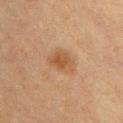workup: imaged on a skin check; not biopsied
patient: female, in their mid-50s
size: ≈4 mm
image-analysis metrics: a footprint of about 8.5 mm², a shape eccentricity near 0.7, and a symmetry-axis asymmetry near 0.25; an average lesion color of about L≈43 a*≈17 b*≈30 (CIELAB), a lesion–skin lightness drop of about 7, and a lesion-to-skin contrast of about 6.5 (normalized; higher = more distinct); border irregularity of about 2.5 on a 0–10 scale, a color-variation rating of about 3/10, and radial color variation of about 1
body site: the front of the torso
lighting: cross-polarized illumination
image source: 15 mm crop, total-body photography